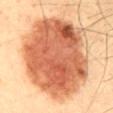Recorded during total-body skin imaging; not selected for excision or biopsy. This image is a 15 mm lesion crop taken from a total-body photograph. From the mid back. Longest diameter approximately 12 mm. A male patient, in their 50s.